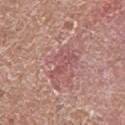notes = imaged on a skin check; not biopsied | imaging modality = ~15 mm crop, total-body skin-cancer survey | lesion size = ~4 mm (longest diameter) | subject = male, approximately 80 years of age | site = the left lower leg | lighting = white-light illumination | automated lesion analysis = border irregularity of about 4.5 on a 0–10 scale, a color-variation rating of about 2.5/10, and radial color variation of about 1.